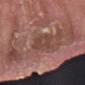Q: Was this lesion biopsied?
A: imaged on a skin check; not biopsied
Q: How was this image acquired?
A: ~15 mm crop, total-body skin-cancer survey
Q: Illumination type?
A: white-light illumination
Q: Automated lesion metrics?
A: a border-irregularity rating of about 2.5/10 and radial color variation of about 1
Q: What is the lesion's diameter?
A: about 3.5 mm
Q: Lesion location?
A: the right forearm
Q: What are the patient's age and sex?
A: male, in their 40s